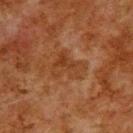follow-up: no biopsy performed (imaged during a skin exam) | automated metrics: an area of roughly 8 mm², an outline eccentricity of about 0.65 (0 = round, 1 = elongated), and a shape-asymmetry score of about 0.55 (0 = symmetric); a border-irregularity rating of about 6.5/10 and a peripheral color-asymmetry measure near 1 | site: the upper back | image: 15 mm crop, total-body photography | lesion diameter: ~4 mm (longest diameter) | patient: male, aged approximately 80 | illumination: cross-polarized illumination.